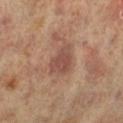Part of a total-body skin-imaging series; this lesion was reviewed on a skin check and was not flagged for biopsy.